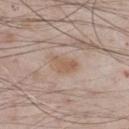imaging modality=total-body-photography crop, ~15 mm field of view | automated metrics=an area of roughly 5 mm² and an eccentricity of roughly 0.8; a lesion color around L≈57 a*≈16 b*≈29 in CIELAB and roughly 7 lightness units darker than nearby skin; a border-irregularity index near 4/10 and a within-lesion color-variation index near 1.5/10; a classifier nevus-likeness of about 5/100 and lesion-presence confidence of about 100/100 | lighting=white-light illumination | site=the front of the torso | lesion size=about 3.5 mm | patient=male, aged 63–67.